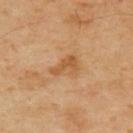follow-up: no biopsy performed (imaged during a skin exam) | patient: male, aged around 50 | size: about 3.5 mm | TBP lesion metrics: a mean CIELAB color near L≈54 a*≈22 b*≈40 and roughly 9 lightness units darker than nearby skin; an automated nevus-likeness rating near 0 out of 100 and lesion-presence confidence of about 100/100 | acquisition: ~15 mm crop, total-body skin-cancer survey | site: the upper back.